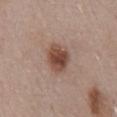The lesion was photographed on a routine skin check and not biopsied; there is no pathology result. A 15 mm close-up tile from a total-body photography series done for melanoma screening. The lesion is on the mid back. A male patient, aged 53 to 57.